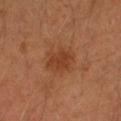This lesion was catalogued during total-body skin photography and was not selected for biopsy.
On the arm.
A male subject aged approximately 40.
A close-up tile cropped from a whole-body skin photograph, about 15 mm across.